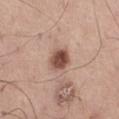{
  "site": "leg",
  "automated_metrics": {
    "eccentricity": 0.6,
    "shape_asymmetry": 0.15,
    "nevus_likeness_0_100": 95,
    "lesion_detection_confidence_0_100": 100
  },
  "patient": {
    "sex": "male",
    "age_approx": 55
  },
  "lesion_size": {
    "long_diameter_mm_approx": 3.0
  },
  "lighting": "white-light",
  "image": {
    "source": "total-body photography crop",
    "field_of_view_mm": 15
  }
}